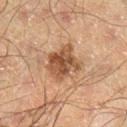Q: Is there a histopathology result?
A: catalogued during a skin exam; not biopsied
Q: Where on the body is the lesion?
A: the right lower leg
Q: What is the lesion's diameter?
A: ≈4 mm
Q: Illumination type?
A: cross-polarized
Q: What did automated image analysis measure?
A: a border-irregularity rating of about 3/10 and a within-lesion color-variation index near 4.5/10
Q: What kind of image is this?
A: total-body-photography crop, ~15 mm field of view
Q: Patient demographics?
A: male, in their 50s